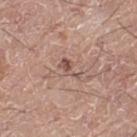Part of a total-body skin-imaging series; this lesion was reviewed on a skin check and was not flagged for biopsy.
Imaged with white-light lighting.
Approximately 3 mm at its widest.
The lesion is located on the left thigh.
A male subject aged approximately 75.
A roughly 15 mm field-of-view crop from a total-body skin photograph.